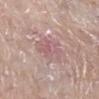follow-up = imaged on a skin check; not biopsied | patient = female, about 65 years old | anatomic site = the right lower leg | size = ~3.5 mm (longest diameter) | TBP lesion metrics = an eccentricity of roughly 0.6 and two-axis asymmetry of about 0.35; a border-irregularity index near 4/10 and a peripheral color-asymmetry measure near 1; a nevus-likeness score of about 0/100 and a lesion-detection confidence of about 85/100 | acquisition = total-body-photography crop, ~15 mm field of view | illumination = white-light illumination.